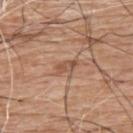• follow-up — imaged on a skin check; not biopsied
• tile lighting — white-light illumination
• diameter — about 3 mm
• image-analysis metrics — a lesion area of about 3.5 mm², an outline eccentricity of about 0.9 (0 = round, 1 = elongated), and two-axis asymmetry of about 0.3
• anatomic site — the back
• patient — male, aged 58 to 62
• acquisition — 15 mm crop, total-body photography